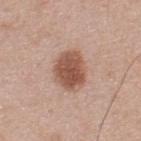Q: Is there a histopathology result?
A: catalogued during a skin exam; not biopsied
Q: Lesion size?
A: ≈4.5 mm
Q: What is the anatomic site?
A: the upper back
Q: Who is the patient?
A: male, in their mid- to late 40s
Q: Illumination type?
A: white-light illumination
Q: What kind of image is this?
A: ~15 mm tile from a whole-body skin photo